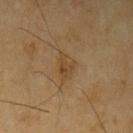<record>
<biopsy_status>not biopsied; imaged during a skin examination</biopsy_status>
<lesion_size>
  <long_diameter_mm_approx>3.0</long_diameter_mm_approx>
</lesion_size>
<lighting>cross-polarized</lighting>
<site>left upper arm</site>
<patient>
  <sex>male</sex>
  <age_approx>70</age_approx>
</patient>
<image>
  <source>total-body photography crop</source>
  <field_of_view_mm>15</field_of_view_mm>
</image>
</record>